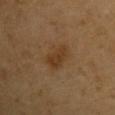Part of a total-body skin-imaging series; this lesion was reviewed on a skin check and was not flagged for biopsy. Measured at roughly 3.5 mm in maximum diameter. A roughly 15 mm field-of-view crop from a total-body skin photograph. The lesion is on the arm. Imaged with cross-polarized lighting. The total-body-photography lesion software estimated an average lesion color of about L≈36 a*≈17 b*≈32 (CIELAB), about 7 CIELAB-L* units darker than the surrounding skin, and a lesion-to-skin contrast of about 7 (normalized; higher = more distinct). And it measured a border-irregularity rating of about 3.5/10 and internal color variation of about 2 on a 0–10 scale. The software also gave an automated nevus-likeness rating near 40 out of 100 and lesion-presence confidence of about 100/100. A male subject aged around 60.